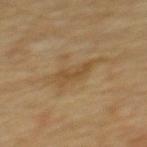The lesion was photographed on a routine skin check and not biopsied; there is no pathology result.
Measured at roughly 5 mm in maximum diameter.
Cropped from a whole-body photographic skin survey; the tile spans about 15 mm.
Automated image analysis of the tile measured a border-irregularity index near 5/10 and a within-lesion color-variation index near 2/10.
A male patient roughly 70 years of age.
Located on the upper back.
This is a cross-polarized tile.